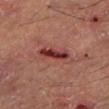biopsy status=imaged on a skin check; not biopsied | acquisition=~15 mm crop, total-body skin-cancer survey | lighting=cross-polarized | lesion diameter=≈4 mm | subject=male, about 55 years old | body site=the left lower leg.